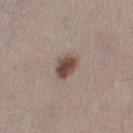{
  "biopsy_status": "not biopsied; imaged during a skin examination",
  "site": "left thigh",
  "automated_metrics": {
    "area_mm2_approx": 5.5,
    "eccentricity": 0.75,
    "shape_asymmetry": 0.2,
    "border_irregularity_0_10": 2.0,
    "color_variation_0_10": 4.0,
    "peripheral_color_asymmetry": 1.0,
    "nevus_likeness_0_100": 100,
    "lesion_detection_confidence_0_100": 100
  },
  "image": {
    "source": "total-body photography crop",
    "field_of_view_mm": 15
  },
  "lighting": "white-light",
  "patient": {
    "sex": "female",
    "age_approx": 40
  },
  "lesion_size": {
    "long_diameter_mm_approx": 3.5
  }
}